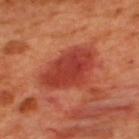workup = imaged on a skin check; not biopsied
illumination = cross-polarized illumination
diameter = about 6.5 mm
acquisition = ~15 mm tile from a whole-body skin photo
subject = male, roughly 50 years of age
anatomic site = the upper back